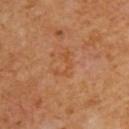Case summary:
* biopsy status: no biopsy performed (imaged during a skin exam)
* image source: total-body-photography crop, ~15 mm field of view
* lighting: cross-polarized
* patient: male, about 60 years old
* body site: the upper back